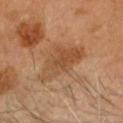| field | value |
|---|---|
| follow-up | no biopsy performed (imaged during a skin exam) |
| subject | male, aged 63 to 67 |
| image source | ~15 mm tile from a whole-body skin photo |
| illumination | cross-polarized |
| location | the head or neck |
| size | about 6 mm |
| image-analysis metrics | a detector confidence of about 100 out of 100 that the crop contains a lesion |